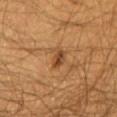Imaged during a routine full-body skin examination; the lesion was not biopsied and no histopathology is available. The lesion is located on the chest. Approximately 2.5 mm at its widest. The tile uses cross-polarized illumination. Automated image analysis of the tile measured an average lesion color of about L≈41 a*≈20 b*≈34 (CIELAB), roughly 10 lightness units darker than nearby skin, and a normalized lesion–skin contrast near 8. The analysis additionally found a border-irregularity rating of about 2.5/10, internal color variation of about 3.5 on a 0–10 scale, and radial color variation of about 1. The patient is a male about 60 years old. A region of skin cropped from a whole-body photographic capture, roughly 15 mm wide.